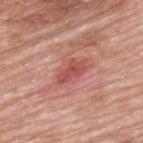{
  "biopsy_status": "not biopsied; imaged during a skin examination",
  "site": "upper back",
  "image": {
    "source": "total-body photography crop",
    "field_of_view_mm": 15
  },
  "lesion_size": {
    "long_diameter_mm_approx": 3.5
  },
  "patient": {
    "sex": "male",
    "age_approx": 80
  },
  "automated_metrics": {
    "area_mm2_approx": 5.0,
    "shape_asymmetry": 0.3,
    "cielab_L": 52,
    "cielab_a": 32,
    "cielab_b": 27,
    "vs_skin_darker_L": 10.0,
    "border_irregularity_0_10": 3.5,
    "color_variation_0_10": 2.0,
    "nevus_likeness_0_100": 0,
    "lesion_detection_confidence_0_100": 100
  },
  "lighting": "white-light"
}